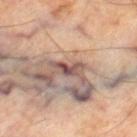The lesion was tiled from a total-body skin photograph and was not biopsied. Located on the leg. The tile uses cross-polarized illumination. A male subject about 70 years old. A region of skin cropped from a whole-body photographic capture, roughly 15 mm wide. Measured at roughly 3.5 mm in maximum diameter.